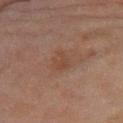| key | value |
|---|---|
| workup | total-body-photography surveillance lesion; no biopsy |
| illumination | cross-polarized illumination |
| patient | female, aged around 60 |
| image | 15 mm crop, total-body photography |
| size | ~2.5 mm (longest diameter) |
| anatomic site | the right thigh |
| TBP lesion metrics | a footprint of about 3.5 mm², an outline eccentricity of about 0.7 (0 = round, 1 = elongated), and two-axis asymmetry of about 0.2; a border-irregularity index near 2/10, a within-lesion color-variation index near 1.5/10, and a peripheral color-asymmetry measure near 0.5; a nevus-likeness score of about 5/100 |